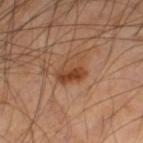No biopsy was performed on this lesion — it was imaged during a full skin examination and was not determined to be concerning. From the right lower leg. Automated tile analysis of the lesion measured a lesion area of about 7.5 mm², an eccentricity of roughly 0.65, and two-axis asymmetry of about 0.3. It also reported a lesion–skin lightness drop of about 9 and a normalized border contrast of about 7.5. The analysis additionally found a border-irregularity rating of about 3/10 and radial color variation of about 3.5. The software also gave an automated nevus-likeness rating near 80 out of 100 and lesion-presence confidence of about 100/100. The subject is a male approximately 50 years of age. Cropped from a whole-body photographic skin survey; the tile spans about 15 mm.